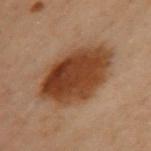The lesion was tiled from a total-body skin photograph and was not biopsied.
The lesion is on the upper back.
Automated image analysis of the tile measured about 13 CIELAB-L* units darker than the surrounding skin and a normalized border contrast of about 11.5. The software also gave a border-irregularity rating of about 1.5/10, a color-variation rating of about 6/10, and a peripheral color-asymmetry measure near 2. The software also gave an automated nevus-likeness rating near 100 out of 100 and lesion-presence confidence of about 100/100.
Imaged with cross-polarized lighting.
A 15 mm close-up tile from a total-body photography series done for melanoma screening.
Measured at roughly 8 mm in maximum diameter.
The patient is a female aged approximately 60.